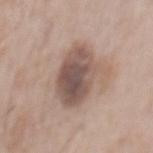| field | value |
|---|---|
| acquisition | ~15 mm tile from a whole-body skin photo |
| lesion diameter | ≈6.5 mm |
| anatomic site | the mid back |
| automated lesion analysis | a lesion color around L≈52 a*≈16 b*≈22 in CIELAB, roughly 13 lightness units darker than nearby skin, and a lesion-to-skin contrast of about 9 (normalized; higher = more distinct); a border-irregularity rating of about 2/10, internal color variation of about 6 on a 0–10 scale, and radial color variation of about 2; a nevus-likeness score of about 10/100 and a detector confidence of about 100 out of 100 that the crop contains a lesion |
| lighting | white-light illumination |
| patient | male, aged 73 to 77 |